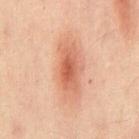No biopsy was performed on this lesion — it was imaged during a full skin examination and was not determined to be concerning. Captured under cross-polarized illumination. The lesion-visualizer software estimated a footprint of about 15 mm², a shape eccentricity near 0.9, and a symmetry-axis asymmetry near 0.2. It also reported roughly 10 lightness units darker than nearby skin and a normalized lesion–skin contrast near 7. It also reported an automated nevus-likeness rating near 95 out of 100 and a detector confidence of about 100 out of 100 that the crop contains a lesion. From the mid back. The subject is a male in their 50s. A close-up tile cropped from a whole-body skin photograph, about 15 mm across.